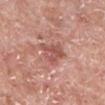The lesion was tiled from a total-body skin photograph and was not biopsied. Approximately 4 mm at its widest. A male patient, aged around 65. A 15 mm close-up tile from a total-body photography series done for melanoma screening. Automated image analysis of the tile measured a footprint of about 7.5 mm² and an eccentricity of roughly 0.7. The software also gave a lesion color around L≈54 a*≈26 b*≈26 in CIELAB, about 10 CIELAB-L* units darker than the surrounding skin, and a normalized lesion–skin contrast near 7. The software also gave an automated nevus-likeness rating near 0 out of 100 and lesion-presence confidence of about 100/100. From the leg.